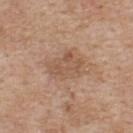Imaged during a routine full-body skin examination; the lesion was not biopsied and no histopathology is available. From the upper back. This image is a 15 mm lesion crop taken from a total-body photograph. A male subject aged approximately 60. The tile uses white-light illumination.